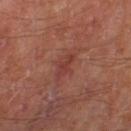The lesion was photographed on a routine skin check and not biopsied; there is no pathology result.
A male patient aged around 70.
The lesion's longest dimension is about 3 mm.
Cropped from a total-body skin-imaging series; the visible field is about 15 mm.
The lesion is on the left thigh.